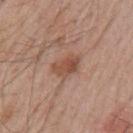Clinical impression: Part of a total-body skin-imaging series; this lesion was reviewed on a skin check and was not flagged for biopsy.